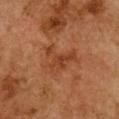Imaged during a routine full-body skin examination; the lesion was not biopsied and no histopathology is available.